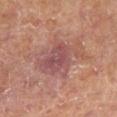Q: Was this lesion biopsied?
A: catalogued during a skin exam; not biopsied
Q: Lesion size?
A: ≈7 mm
Q: Lesion location?
A: the left lower leg
Q: What are the patient's age and sex?
A: male, approximately 65 years of age
Q: What kind of image is this?
A: 15 mm crop, total-body photography